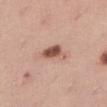Assessment:
Part of a total-body skin-imaging series; this lesion was reviewed on a skin check and was not flagged for biopsy.
Context:
This is a white-light tile. Cropped from a whole-body photographic skin survey; the tile spans about 15 mm. Measured at roughly 4 mm in maximum diameter. An algorithmic analysis of the crop reported roughly 14 lightness units darker than nearby skin and a normalized lesion–skin contrast near 9.5. The subject is a female about 45 years old. On the right thigh.